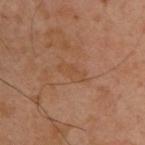Notes:
• notes: imaged on a skin check; not biopsied
• image-analysis metrics: an area of roughly 4 mm²; an average lesion color of about L≈48 a*≈21 b*≈33 (CIELAB) and a lesion-to-skin contrast of about 4.5 (normalized; higher = more distinct); border irregularity of about 4 on a 0–10 scale, internal color variation of about 1 on a 0–10 scale, and radial color variation of about 0; an automated nevus-likeness rating near 0 out of 100 and lesion-presence confidence of about 100/100
• subject: male, aged 38–42
• diameter: about 3 mm
• image: ~15 mm tile from a whole-body skin photo
• lighting: cross-polarized illumination
• anatomic site: the upper back